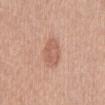Q: Was a biopsy performed?
A: catalogued during a skin exam; not biopsied
Q: How was this image acquired?
A: 15 mm crop, total-body photography
Q: Patient demographics?
A: female, aged 53–57
Q: What is the lesion's diameter?
A: ~4 mm (longest diameter)
Q: Lesion location?
A: the front of the torso
Q: How was the tile lit?
A: white-light